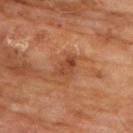| field | value |
|---|---|
| follow-up | imaged on a skin check; not biopsied |
| body site | the chest |
| subject | male, aged approximately 60 |
| image | total-body-photography crop, ~15 mm field of view |
| automated metrics | a border-irregularity index near 3.5/10, a within-lesion color-variation index near 4/10, and a peripheral color-asymmetry measure near 1.5; an automated nevus-likeness rating near 5 out of 100 and a lesion-detection confidence of about 100/100 |